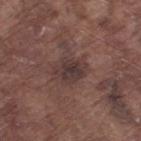<tbp_lesion>
<biopsy_status>not biopsied; imaged during a skin examination</biopsy_status>
<automated_metrics>
  <cielab_L>35</cielab_L>
  <cielab_a>16</cielab_a>
  <cielab_b>17</cielab_b>
  <vs_skin_contrast_norm>8.0</vs_skin_contrast_norm>
  <border_irregularity_0_10>2.5</border_irregularity_0_10>
  <color_variation_0_10>3.5</color_variation_0_10>
  <peripheral_color_asymmetry>1.0</peripheral_color_asymmetry>
</automated_metrics>
<image>
  <source>total-body photography crop</source>
  <field_of_view_mm>15</field_of_view_mm>
</image>
<patient>
  <sex>male</sex>
  <age_approx>75</age_approx>
</patient>
<lighting>white-light</lighting>
<site>right thigh</site>
<lesion_size>
  <long_diameter_mm_approx>4.0</long_diameter_mm_approx>
</lesion_size>
</tbp_lesion>